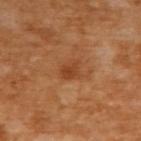biopsy_status: not biopsied; imaged during a skin examination
patient:
  sex: female
  age_approx: 55
site: upper back
image:
  source: total-body photography crop
  field_of_view_mm: 15